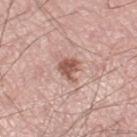– notes · imaged on a skin check; not biopsied
– anatomic site · the left thigh
– lesion size · about 3 mm
– subject · male, aged 68–72
– image · total-body-photography crop, ~15 mm field of view